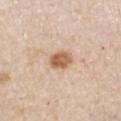Part of a total-body skin-imaging series; this lesion was reviewed on a skin check and was not flagged for biopsy.
From the front of the torso.
A female patient, about 45 years old.
A lesion tile, about 15 mm wide, cut from a 3D total-body photograph.
Longest diameter approximately 3 mm.